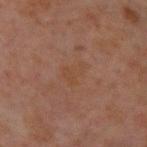<case>
  <biopsy_status>not biopsied; imaged during a skin examination</biopsy_status>
  <patient>
    <sex>male</sex>
    <age_approx>30</age_approx>
  </patient>
  <site>arm</site>
  <lighting>cross-polarized</lighting>
  <image>
    <source>total-body photography crop</source>
    <field_of_view_mm>15</field_of_view_mm>
  </image>
  <lesion_size>
    <long_diameter_mm_approx>3.5</long_diameter_mm_approx>
  </lesion_size>
</case>